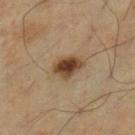The lesion was tiled from a total-body skin photograph and was not biopsied. The lesion is located on the leg. A close-up tile cropped from a whole-body skin photograph, about 15 mm across. A male patient, aged 68 to 72. Automated image analysis of the tile measured an area of roughly 7 mm², a shape eccentricity near 0.65, and a shape-asymmetry score of about 0.2 (0 = symmetric). The analysis additionally found an average lesion color of about L≈40 a*≈16 b*≈29 (CIELAB) and a lesion–skin lightness drop of about 15. The software also gave an automated nevus-likeness rating near 100 out of 100 and lesion-presence confidence of about 100/100. Measured at roughly 3.5 mm in maximum diameter.